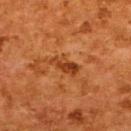{
  "biopsy_status": "not biopsied; imaged during a skin examination",
  "patient": {
    "sex": "female",
    "age_approx": 50
  },
  "image": {
    "source": "total-body photography crop",
    "field_of_view_mm": 15
  },
  "site": "upper back",
  "lighting": "cross-polarized"
}